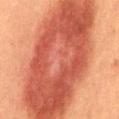Impression:
Captured during whole-body skin photography for melanoma surveillance; the lesion was not biopsied.
Background:
The tile uses cross-polarized illumination. A 15 mm close-up tile from a total-body photography series done for melanoma screening. A male patient, roughly 40 years of age. From the mid back. About 18.5 mm across.